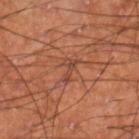{"biopsy_status": "not biopsied; imaged during a skin examination", "site": "right lower leg", "lesion_size": {"long_diameter_mm_approx": 2.5}, "image": {"source": "total-body photography crop", "field_of_view_mm": 15}, "patient": {"sex": "male", "age_approx": 60}, "automated_metrics": {"area_mm2_approx": 2.5, "cielab_L": 43, "cielab_a": 24, "cielab_b": 29, "vs_skin_darker_L": 7.0, "vs_skin_contrast_norm": 6.0, "nevus_likeness_0_100": 0, "lesion_detection_confidence_0_100": 55}, "lighting": "cross-polarized"}